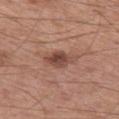No biopsy was performed on this lesion — it was imaged during a full skin examination and was not determined to be concerning.
Captured under white-light illumination.
Located on the leg.
A male subject, in their mid-50s.
A close-up tile cropped from a whole-body skin photograph, about 15 mm across.
Longest diameter approximately 4 mm.